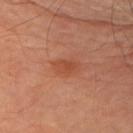The lesion was tiled from a total-body skin photograph and was not biopsied.
A roughly 15 mm field-of-view crop from a total-body skin photograph.
From the left upper arm.